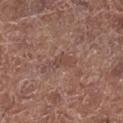Q: Was a biopsy performed?
A: total-body-photography surveillance lesion; no biopsy
Q: Patient demographics?
A: female, aged 48 to 52
Q: What did automated image analysis measure?
A: border irregularity of about 4.5 on a 0–10 scale and a color-variation rating of about 1.5/10; a classifier nevus-likeness of about 0/100
Q: What is the imaging modality?
A: total-body-photography crop, ~15 mm field of view
Q: Lesion location?
A: the leg
Q: Illumination type?
A: white-light